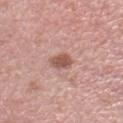image-analysis metrics — a border-irregularity rating of about 2/10 and a peripheral color-asymmetry measure near 0.5; an automated nevus-likeness rating near 80 out of 100 and a lesion-detection confidence of about 100/100
tile lighting — white-light illumination
patient — female, aged approximately 40
body site — the right lower leg
image — 15 mm crop, total-body photography